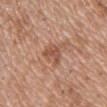This lesion was catalogued during total-body skin photography and was not selected for biopsy.
Located on the chest.
A region of skin cropped from a whole-body photographic capture, roughly 15 mm wide.
The patient is a male aged 48 to 52.